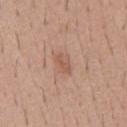Q: Is there a histopathology result?
A: catalogued during a skin exam; not biopsied
Q: Who is the patient?
A: male, aged 38–42
Q: What is the imaging modality?
A: ~15 mm tile from a whole-body skin photo
Q: Automated lesion metrics?
A: a mean CIELAB color near L≈56 a*≈21 b*≈29 and roughly 7 lightness units darker than nearby skin; border irregularity of about 2 on a 0–10 scale, internal color variation of about 2 on a 0–10 scale, and radial color variation of about 0.5; a nevus-likeness score of about 5/100
Q: What is the anatomic site?
A: the chest